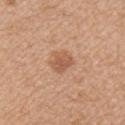Clinical impression:
The lesion was tiled from a total-body skin photograph and was not biopsied.
Background:
The patient is a female aged around 45. Imaged with white-light lighting. Approximately 2.5 mm at its widest. A 15 mm crop from a total-body photograph taken for skin-cancer surveillance. From the front of the torso.